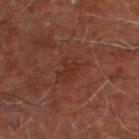follow-up: catalogued during a skin exam; not biopsied
site: the right leg
subject: male, approximately 60 years of age
imaging modality: ~15 mm tile from a whole-body skin photo